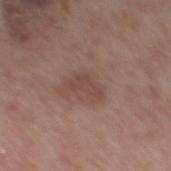No biopsy was performed on this lesion — it was imaged during a full skin examination and was not determined to be concerning. The recorded lesion diameter is about 3 mm. A male subject, aged approximately 65. A roughly 15 mm field-of-view crop from a total-body skin photograph. On the back. The total-body-photography lesion software estimated a lesion area of about 4 mm², an eccentricity of roughly 0.85, and a shape-asymmetry score of about 0.55 (0 = symmetric). The analysis additionally found roughly 7 lightness units darker than nearby skin. The software also gave lesion-presence confidence of about 100/100. This is a white-light tile.